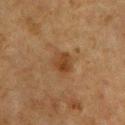Assessment: Imaged during a routine full-body skin examination; the lesion was not biopsied and no histopathology is available. Image and clinical context: A 15 mm crop from a total-body photograph taken for skin-cancer surveillance. The patient is a male aged 58 to 62. Imaged with cross-polarized lighting. From the chest. The recorded lesion diameter is about 2.5 mm.